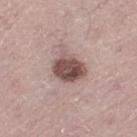The lesion was tiled from a total-body skin photograph and was not biopsied. The lesion is located on the left thigh. The patient is a male aged approximately 60. A lesion tile, about 15 mm wide, cut from a 3D total-body photograph.